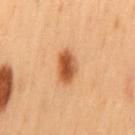automated_metrics:
  border_irregularity_0_10: 2.0
  color_variation_0_10: 5.0
  peripheral_color_asymmetry: 1.5
  nevus_likeness_0_100: 100
  lesion_detection_confidence_0_100: 100
patient:
  sex: male
  age_approx: 55
site: mid back
lighting: cross-polarized
image:
  source: total-body photography crop
  field_of_view_mm: 15